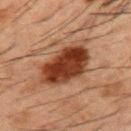This lesion was catalogued during total-body skin photography and was not selected for biopsy. The recorded lesion diameter is about 6.5 mm. The subject is a male aged 48 to 52. The lesion is on the back. This image is a 15 mm lesion crop taken from a total-body photograph.